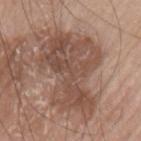Findings:
– site — the arm
– patient — male, about 75 years old
– imaging modality — ~15 mm crop, total-body skin-cancer survey
– illumination — white-light illumination
– lesion diameter — about 10 mm